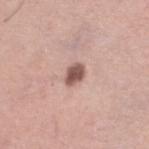follow-up: imaged on a skin check; not biopsied
image: ~15 mm tile from a whole-body skin photo
subject: female, aged around 40
location: the left thigh
lesion size: ≈2.5 mm
automated lesion analysis: an outline eccentricity of about 0.75 (0 = round, 1 = elongated) and two-axis asymmetry of about 0.3; a mean CIELAB color near L≈54 a*≈21 b*≈24, roughly 16 lightness units darker than nearby skin, and a normalized border contrast of about 10.5; a classifier nevus-likeness of about 70/100 and lesion-presence confidence of about 100/100